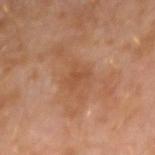Impression: Captured during whole-body skin photography for melanoma surveillance; the lesion was not biopsied.